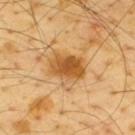biopsy status — no biopsy performed (imaged during a skin exam)
location — the back
imaging modality — ~15 mm tile from a whole-body skin photo
subject — male, in their mid- to late 60s
TBP lesion metrics — a lesion area of about 11 mm², an outline eccentricity of about 0.75 (0 = round, 1 = elongated), and a symmetry-axis asymmetry near 0.15; roughly 13 lightness units darker than nearby skin; a border-irregularity index near 2/10, a color-variation rating of about 5/10, and peripheral color asymmetry of about 1; a classifier nevus-likeness of about 40/100 and a detector confidence of about 100 out of 100 that the crop contains a lesion
size — ≈4.5 mm